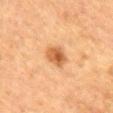The lesion was photographed on a routine skin check and not biopsied; there is no pathology result.
From the mid back.
This image is a 15 mm lesion crop taken from a total-body photograph.
A male patient, approximately 85 years of age or older.
The lesion-visualizer software estimated a lesion area of about 5.5 mm² and a shape-asymmetry score of about 0.2 (0 = symmetric). And it measured an average lesion color of about L≈52 a*≈23 b*≈38 (CIELAB) and a normalized lesion–skin contrast near 8.5. And it measured internal color variation of about 5 on a 0–10 scale and peripheral color asymmetry of about 2. The analysis additionally found an automated nevus-likeness rating near 95 out of 100 and lesion-presence confidence of about 100/100.
The recorded lesion diameter is about 3 mm.
Captured under cross-polarized illumination.